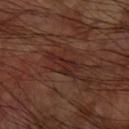The lesion was photographed on a routine skin check and not biopsied; there is no pathology result. The subject is a male aged 58–62. From the right upper arm. A 15 mm close-up extracted from a 3D total-body photography capture.